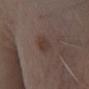No biopsy was performed on this lesion — it was imaged during a full skin examination and was not determined to be concerning.
Longest diameter approximately 2.5 mm.
From the left lower leg.
Cropped from a total-body skin-imaging series; the visible field is about 15 mm.
The lesion-visualizer software estimated a border-irregularity index near 1.5/10, a within-lesion color-variation index near 2/10, and radial color variation of about 0.5. The analysis additionally found an automated nevus-likeness rating near 30 out of 100 and a detector confidence of about 100 out of 100 that the crop contains a lesion.
A male subject about 70 years old.
The tile uses white-light illumination.